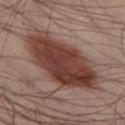Assessment:
Part of a total-body skin-imaging series; this lesion was reviewed on a skin check and was not flagged for biopsy.
Clinical summary:
Measured at roughly 10 mm in maximum diameter. On the left thigh. Imaged with cross-polarized lighting. A roughly 15 mm field-of-view crop from a total-body skin photograph. A male patient aged 38 to 42.